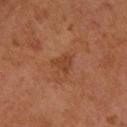Impression: Captured during whole-body skin photography for melanoma surveillance; the lesion was not biopsied. Image and clinical context: A female subject, in their 60s. Measured at roughly 2.5 mm in maximum diameter. An algorithmic analysis of the crop reported an area of roughly 4 mm² and a shape-asymmetry score of about 0.45 (0 = symmetric). It also reported a border-irregularity index near 4/10, internal color variation of about 1.5 on a 0–10 scale, and radial color variation of about 0.5. This is a cross-polarized tile. Cropped from a whole-body photographic skin survey; the tile spans about 15 mm. The lesion is on the left forearm.